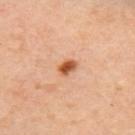  biopsy_status: not biopsied; imaged during a skin examination
  image:
    source: total-body photography crop
    field_of_view_mm: 15
  automated_metrics:
    eccentricity: 0.75
    cielab_L: 56
    cielab_a: 27
    cielab_b: 39
    vs_skin_darker_L: 15.0
    vs_skin_contrast_norm: 10.5
    border_irregularity_0_10: 1.5
    peripheral_color_asymmetry: 1.0
  patient:
    sex: female
    age_approx: 40
  site: upper back